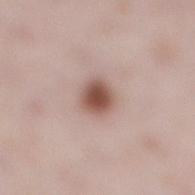{
  "biopsy_status": "not biopsied; imaged during a skin examination",
  "site": "right lower leg",
  "patient": {
    "sex": "female",
    "age_approx": 30
  },
  "image": {
    "source": "total-body photography crop",
    "field_of_view_mm": 15
  },
  "automated_metrics": {
    "eccentricity": 0.6,
    "shape_asymmetry": 0.15,
    "peripheral_color_asymmetry": 1.0,
    "nevus_likeness_0_100": 100,
    "lesion_detection_confidence_0_100": 100
  },
  "lighting": "white-light"
}